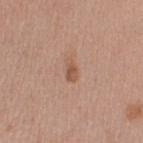biopsy_status: not biopsied; imaged during a skin examination
image:
  source: total-body photography crop
  field_of_view_mm: 15
lighting: white-light
site: left upper arm
patient:
  sex: female
  age_approx: 40
automated_metrics:
  eccentricity: 0.85
  shape_asymmetry: 0.3
  nevus_likeness_0_100: 40
  lesion_detection_confidence_0_100: 100